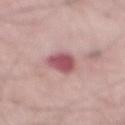Imaged during a routine full-body skin examination; the lesion was not biopsied and no histopathology is available.
The recorded lesion diameter is about 4 mm.
The lesion is located on the mid back.
A male subject, aged around 65.
Imaged with white-light lighting.
A close-up tile cropped from a whole-body skin photograph, about 15 mm across.